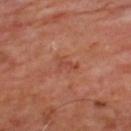Assessment: Part of a total-body skin-imaging series; this lesion was reviewed on a skin check and was not flagged for biopsy. Acquisition and patient details: A 15 mm crop from a total-body photograph taken for skin-cancer surveillance. Imaged with cross-polarized lighting. The lesion is on the back. Longest diameter approximately 3 mm. A male subject, aged 63 to 67.